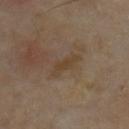{"biopsy_status": "not biopsied; imaged during a skin examination", "image": {"source": "total-body photography crop", "field_of_view_mm": 15}, "lesion_size": {"long_diameter_mm_approx": 4.0}, "automated_metrics": {"area_mm2_approx": 4.5, "eccentricity": 0.95, "shape_asymmetry": 0.4, "cielab_L": 40, "cielab_a": 14, "cielab_b": 29, "vs_skin_darker_L": 6.0, "vs_skin_contrast_norm": 6.5}, "patient": {"sex": "male", "age_approx": 65}, "lighting": "cross-polarized", "site": "front of the torso"}